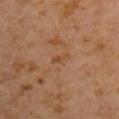follow-up — no biopsy performed (imaged during a skin exam)
location — the right upper arm
lighting — cross-polarized
automated metrics — an outline eccentricity of about 0.9 (0 = round, 1 = elongated) and a symmetry-axis asymmetry near 0.35; an average lesion color of about L≈43 a*≈19 b*≈33 (CIELAB) and a lesion-to-skin contrast of about 5.5 (normalized; higher = more distinct); a border-irregularity rating of about 4/10 and peripheral color asymmetry of about 0; an automated nevus-likeness rating near 0 out of 100 and lesion-presence confidence of about 100/100
lesion diameter — ≈2.5 mm
subject — male, approximately 60 years of age
imaging modality — 15 mm crop, total-body photography